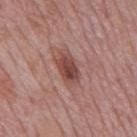Imaged during a routine full-body skin examination; the lesion was not biopsied and no histopathology is available.
The lesion is located on the mid back.
A male subject, in their mid- to late 70s.
The recorded lesion diameter is about 3.5 mm.
The tile uses white-light illumination.
A close-up tile cropped from a whole-body skin photograph, about 15 mm across.